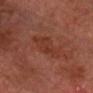Q: Is there a histopathology result?
A: total-body-photography surveillance lesion; no biopsy
Q: What are the patient's age and sex?
A: male, aged 73–77
Q: What kind of image is this?
A: ~15 mm tile from a whole-body skin photo
Q: Where on the body is the lesion?
A: the chest
Q: What lighting was used for the tile?
A: cross-polarized illumination
Q: How large is the lesion?
A: ~5.5 mm (longest diameter)